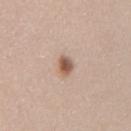Captured during whole-body skin photography for melanoma surveillance; the lesion was not biopsied. The tile uses white-light illumination. The lesion is on the right upper arm. A lesion tile, about 15 mm wide, cut from a 3D total-body photograph. About 2.5 mm across. A female subject, roughly 25 years of age.